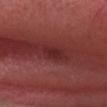follow-up = total-body-photography surveillance lesion; no biopsy | location = the head or neck | diameter = ≈3 mm | automated metrics = an average lesion color of about L≈28 a*≈28 b*≈22 (CIELAB) and a normalized lesion–skin contrast near 7; a nevus-likeness score of about 0/100 and a lesion-detection confidence of about 80/100 | subject = male, about 65 years old | illumination = white-light illumination | image source = 15 mm crop, total-body photography.